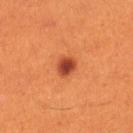Recorded during total-body skin imaging; not selected for excision or biopsy. A female patient, in their 30s. This is a cross-polarized tile. Automated image analysis of the tile measured an average lesion color of about L≈44 a*≈33 b*≈37 (CIELAB) and a lesion-to-skin contrast of about 10.5 (normalized; higher = more distinct). And it measured an automated nevus-likeness rating near 100 out of 100 and a lesion-detection confidence of about 100/100. The recorded lesion diameter is about 2.5 mm. A 15 mm close-up extracted from a 3D total-body photography capture. On the right lower leg.